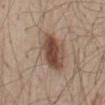  biopsy_status: not biopsied; imaged during a skin examination
  image:
    source: total-body photography crop
    field_of_view_mm: 15
  site: mid back
  patient:
    sex: male
    age_approx: 50